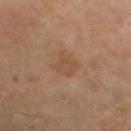{"biopsy_status": "not biopsied; imaged during a skin examination", "image": {"source": "total-body photography crop", "field_of_view_mm": 15}, "site": "right thigh", "patient": {"sex": "male", "age_approx": 60}}